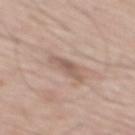{"biopsy_status": "not biopsied; imaged during a skin examination", "automated_metrics": {"border_irregularity_0_10": 4.0, "color_variation_0_10": 2.5, "peripheral_color_asymmetry": 1.0, "nevus_likeness_0_100": 0, "lesion_detection_confidence_0_100": 95}, "lesion_size": {"long_diameter_mm_approx": 4.0}, "image": {"source": "total-body photography crop", "field_of_view_mm": 15}, "lighting": "white-light", "patient": {"sex": "male", "age_approx": 65}, "site": "mid back"}